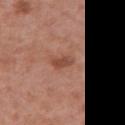<record>
  <image>
    <source>total-body photography crop</source>
    <field_of_view_mm>15</field_of_view_mm>
  </image>
  <patient>
    <sex>female</sex>
    <age_approx>40</age_approx>
  </patient>
  <site>chest</site>
</record>